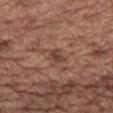Captured during whole-body skin photography for melanoma surveillance; the lesion was not biopsied. Captured under white-light illumination. Automated image analysis of the tile measured a footprint of about 3.5 mm², an outline eccentricity of about 0.85 (0 = round, 1 = elongated), and two-axis asymmetry of about 0.3. The software also gave a lesion color around L≈43 a*≈20 b*≈26 in CIELAB, a lesion–skin lightness drop of about 8, and a normalized lesion–skin contrast near 6.5. The lesion is on the mid back. Measured at roughly 3 mm in maximum diameter. A lesion tile, about 15 mm wide, cut from a 3D total-body photograph. A female patient, roughly 75 years of age.